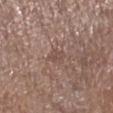Case summary:
• notes: total-body-photography surveillance lesion; no biopsy
• location: the left lower leg
• patient: female, aged 73 to 77
• size: about 2.5 mm
• imaging modality: ~15 mm crop, total-body skin-cancer survey
• lighting: white-light illumination